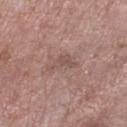Part of a total-body skin-imaging series; this lesion was reviewed on a skin check and was not flagged for biopsy. The lesion is on the left lower leg. The recorded lesion diameter is about 3.5 mm. A lesion tile, about 15 mm wide, cut from a 3D total-body photograph. Automated image analysis of the tile measured an eccentricity of roughly 0.85 and two-axis asymmetry of about 0.6. It also reported a border-irregularity index near 6.5/10, a color-variation rating of about 1.5/10, and radial color variation of about 0.5. The patient is a female in their mid-70s. Imaged with white-light lighting.